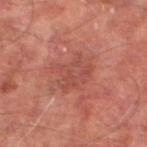The tile uses cross-polarized illumination. Located on the left lower leg. A 15 mm close-up tile from a total-body photography series done for melanoma screening. A male subject about 65 years old. The lesion's longest dimension is about 4.5 mm.